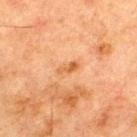  biopsy_status: not biopsied; imaged during a skin examination
  patient:
    sex: male
    age_approx: 70
  lighting: cross-polarized
  lesion_size:
    long_diameter_mm_approx: 2.5
  automated_metrics:
    area_mm2_approx: 2.5
    eccentricity: 0.9
    shape_asymmetry: 0.25
  image:
    source: total-body photography crop
    field_of_view_mm: 15
  site: upper back